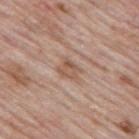This lesion was catalogued during total-body skin photography and was not selected for biopsy. Automated tile analysis of the lesion measured a footprint of about 4.5 mm² and an eccentricity of roughly 0.65. The software also gave a border-irregularity rating of about 3/10, internal color variation of about 4 on a 0–10 scale, and radial color variation of about 1.5. And it measured a classifier nevus-likeness of about 0/100. A lesion tile, about 15 mm wide, cut from a 3D total-body photograph. Captured under white-light illumination. The patient is a male aged 58–62. Approximately 3 mm at its widest. From the mid back.